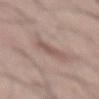Imaged during a routine full-body skin examination; the lesion was not biopsied and no histopathology is available. Cropped from a total-body skin-imaging series; the visible field is about 15 mm. About 3.5 mm across. The patient is a male aged approximately 55. The tile uses white-light illumination. On the abdomen.